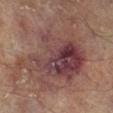notes — total-body-photography surveillance lesion; no biopsy
patient — male, aged around 65
body site — the right lower leg
diameter — about 11.5 mm
lighting — cross-polarized
image-analysis metrics — an area of roughly 60 mm² and a shape-asymmetry score of about 0.35 (0 = symmetric); a nevus-likeness score of about 0/100
image — 15 mm crop, total-body photography